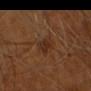biopsy status: total-body-photography surveillance lesion; no biopsy
automated metrics: a border-irregularity index near 3/10, a color-variation rating of about 1.5/10, and radial color variation of about 0.5; a nevus-likeness score of about 10/100 and lesion-presence confidence of about 100/100
imaging modality: ~15 mm crop, total-body skin-cancer survey
patient: male, aged around 55
diameter: about 3 mm
anatomic site: the head or neck